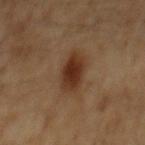Part of a total-body skin-imaging series; this lesion was reviewed on a skin check and was not flagged for biopsy. A roughly 15 mm field-of-view crop from a total-body skin photograph. This is a cross-polarized tile. On the back. A male patient, aged 58 to 62.